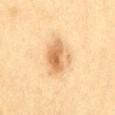Notes:
* notes: no biopsy performed (imaged during a skin exam)
* subject: female, aged 28 to 32
* lesion diameter: about 5 mm
* TBP lesion metrics: a lesion color around L≈64 a*≈18 b*≈39 in CIELAB, roughly 12 lightness units darker than nearby skin, and a lesion-to-skin contrast of about 7.5 (normalized; higher = more distinct); a border-irregularity rating of about 2.5/10 and internal color variation of about 7 on a 0–10 scale; a classifier nevus-likeness of about 90/100 and a lesion-detection confidence of about 100/100
* illumination: cross-polarized
* imaging modality: 15 mm crop, total-body photography
* site: the abdomen